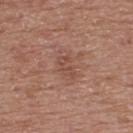No biopsy was performed on this lesion — it was imaged during a full skin examination and was not determined to be concerning.
A male subject, aged approximately 55.
The lesion-visualizer software estimated an average lesion color of about L≈48 a*≈22 b*≈27 (CIELAB), about 7 CIELAB-L* units darker than the surrounding skin, and a normalized border contrast of about 5.5. The analysis additionally found a border-irregularity rating of about 5/10, internal color variation of about 0.5 on a 0–10 scale, and a peripheral color-asymmetry measure near 0.
A 15 mm crop from a total-body photograph taken for skin-cancer surveillance.
Approximately 3 mm at its widest.
Located on the upper back.